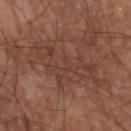This lesion was catalogued during total-body skin photography and was not selected for biopsy.
This is a cross-polarized tile.
The patient is a male in their mid-60s.
On the left thigh.
About 10 mm across.
A 15 mm crop from a total-body photograph taken for skin-cancer surveillance.